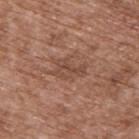Q: Was this lesion biopsied?
A: catalogued during a skin exam; not biopsied
Q: What lighting was used for the tile?
A: white-light illumination
Q: How was this image acquired?
A: ~15 mm tile from a whole-body skin photo
Q: What is the lesion's diameter?
A: about 3.5 mm
Q: Who is the patient?
A: male, in their mid- to late 70s
Q: What is the anatomic site?
A: the back